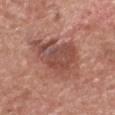{"biopsy_status": "not biopsied; imaged during a skin examination", "image": {"source": "total-body photography crop", "field_of_view_mm": 15}, "automated_metrics": {"area_mm2_approx": 24.0, "eccentricity": 0.4, "shape_asymmetry": 0.3, "cielab_L": 49, "cielab_a": 24, "cielab_b": 26, "vs_skin_contrast_norm": 7.0, "border_irregularity_0_10": 5.0, "color_variation_0_10": 5.5, "peripheral_color_asymmetry": 1.5, "nevus_likeness_0_100": 45, "lesion_detection_confidence_0_100": 100}, "lighting": "white-light", "lesion_size": {"long_diameter_mm_approx": 6.0}, "site": "head or neck", "patient": {"sex": "male", "age_approx": 60}}